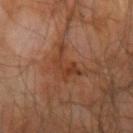Q: What kind of image is this?
A: 15 mm crop, total-body photography
Q: What is the anatomic site?
A: the right upper arm
Q: How large is the lesion?
A: ≈4 mm
Q: What are the patient's age and sex?
A: male, in their mid-60s
Q: What did automated image analysis measure?
A: a nevus-likeness score of about 0/100 and a lesion-detection confidence of about 95/100